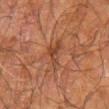{
  "biopsy_status": "not biopsied; imaged during a skin examination",
  "image": {
    "source": "total-body photography crop",
    "field_of_view_mm": 15
  },
  "lesion_size": {
    "long_diameter_mm_approx": 4.0
  },
  "automated_metrics": {
    "border_irregularity_0_10": 6.0,
    "color_variation_0_10": 3.5,
    "peripheral_color_asymmetry": 1.0
  },
  "site": "right forearm",
  "patient": {
    "sex": "male",
    "age_approx": 70
  }
}